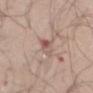A male patient, approximately 40 years of age. Located on the left upper arm. A close-up tile cropped from a whole-body skin photograph, about 15 mm across.